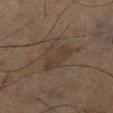| feature | finding |
|---|---|
| workup | imaged on a skin check; not biopsied |
| imaging modality | ~15 mm tile from a whole-body skin photo |
| location | the leg |
| patient | male, aged around 65 |